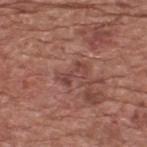Notes:
* biopsy status · imaged on a skin check; not biopsied
* location · the upper back
* imaging modality · ~15 mm crop, total-body skin-cancer survey
* subject · male, in their mid-70s